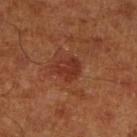No biopsy was performed on this lesion — it was imaged during a full skin examination and was not determined to be concerning.